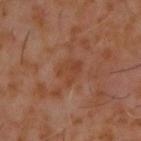A 15 mm crop from a total-body photograph taken for skin-cancer surveillance.
On the upper back.
The tile uses cross-polarized illumination.
The recorded lesion diameter is about 3 mm.
The total-body-photography lesion software estimated a lesion area of about 6 mm² and an outline eccentricity of about 0.55 (0 = round, 1 = elongated). The software also gave a mean CIELAB color near L≈39 a*≈22 b*≈30. And it measured a within-lesion color-variation index near 3/10 and radial color variation of about 1. The software also gave a classifier nevus-likeness of about 0/100 and a detector confidence of about 100 out of 100 that the crop contains a lesion.
A male patient, aged 58–62.